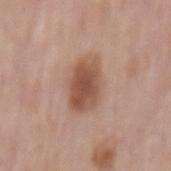follow-up = total-body-photography surveillance lesion; no biopsy
tile lighting = white-light illumination
site = the mid back
size = about 5 mm
patient = male, about 75 years old
image = total-body-photography crop, ~15 mm field of view